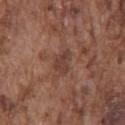Findings:
* biopsy status · catalogued during a skin exam; not biopsied
* subject · male, approximately 75 years of age
* site · the chest
* lighting · white-light
* image-analysis metrics · a footprint of about 5 mm², an outline eccentricity of about 0.8 (0 = round, 1 = elongated), and two-axis asymmetry of about 0.35; a lesion color around L≈40 a*≈20 b*≈25 in CIELAB, a lesion–skin lightness drop of about 8, and a normalized lesion–skin contrast near 6.5; a border-irregularity index near 3.5/10, a within-lesion color-variation index near 3/10, and a peripheral color-asymmetry measure near 1; a nevus-likeness score of about 0/100 and lesion-presence confidence of about 95/100
* size · ≈3.5 mm
* image · ~15 mm crop, total-body skin-cancer survey